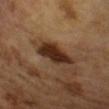Part of a total-body skin-imaging series; this lesion was reviewed on a skin check and was not flagged for biopsy.